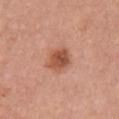biopsy_status: not biopsied; imaged during a skin examination
lighting: white-light
image:
  source: total-body photography crop
  field_of_view_mm: 15
site: right upper arm
patient:
  sex: female
  age_approx: 60